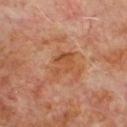{
  "biopsy_status": "not biopsied; imaged during a skin examination",
  "image": {
    "source": "total-body photography crop",
    "field_of_view_mm": 15
  },
  "site": "chest",
  "patient": {
    "sex": "male",
    "age_approx": 65
  },
  "lighting": "cross-polarized",
  "lesion_size": {
    "long_diameter_mm_approx": 3.5
  },
  "automated_metrics": {
    "cielab_L": 49,
    "cielab_a": 24,
    "cielab_b": 36,
    "vs_skin_darker_L": 7.0,
    "vs_skin_contrast_norm": 6.0
  }
}